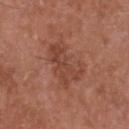Q: Lesion location?
A: the head or neck
Q: Who is the patient?
A: male, aged 48 to 52
Q: How was this image acquired?
A: ~15 mm tile from a whole-body skin photo
Q: How was the tile lit?
A: white-light illumination